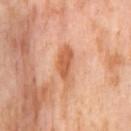Impression:
Recorded during total-body skin imaging; not selected for excision or biopsy.
Image and clinical context:
The lesion is on the right thigh. A female patient, about 55 years old. This image is a 15 mm lesion crop taken from a total-body photograph. Longest diameter approximately 5.5 mm. This is a cross-polarized tile.